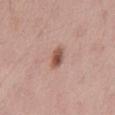Part of a total-body skin-imaging series; this lesion was reviewed on a skin check and was not flagged for biopsy. A male patient, about 55 years old. From the mid back. Automated image analysis of the tile measured a footprint of about 4 mm² and an eccentricity of roughly 0.75. It also reported peripheral color asymmetry of about 2. A region of skin cropped from a whole-body photographic capture, roughly 15 mm wide.